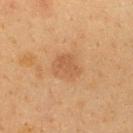Part of a total-body skin-imaging series; this lesion was reviewed on a skin check and was not flagged for biopsy. The lesion is located on the upper back. A female subject, roughly 40 years of age. Imaged with cross-polarized lighting. A roughly 15 mm field-of-view crop from a total-body skin photograph. The lesion's longest dimension is about 3.5 mm.